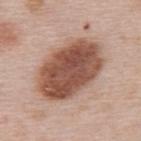Case summary:
• biopsy status: imaged on a skin check; not biopsied
• illumination: white-light
• subject: female, aged approximately 65
• image: ~15 mm tile from a whole-body skin photo
• location: the upper back